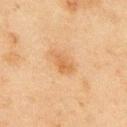Imaged during a routine full-body skin examination; the lesion was not biopsied and no histopathology is available. Imaged with cross-polarized lighting. The lesion-visualizer software estimated a lesion area of about 5 mm² and an eccentricity of roughly 0.8. The software also gave a detector confidence of about 100 out of 100 that the crop contains a lesion. A roughly 15 mm field-of-view crop from a total-body skin photograph. The lesion is on the back. A male subject, approximately 45 years of age.